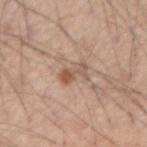| key | value |
|---|---|
| workup | no biopsy performed (imaged during a skin exam) |